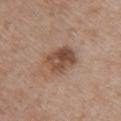Q: Was a biopsy performed?
A: no biopsy performed (imaged during a skin exam)
Q: Lesion size?
A: ~4 mm (longest diameter)
Q: Illumination type?
A: white-light illumination
Q: Lesion location?
A: the upper back
Q: What kind of image is this?
A: ~15 mm tile from a whole-body skin photo
Q: What are the patient's age and sex?
A: female, approximately 50 years of age